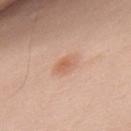subject: male, about 70 years old | lighting: white-light | location: the upper back | lesion size: about 3 mm | image source: 15 mm crop, total-body photography | image-analysis metrics: an area of roughly 4.5 mm², an outline eccentricity of about 0.75 (0 = round, 1 = elongated), and a symmetry-axis asymmetry near 0.2; a border-irregularity index near 2/10 and internal color variation of about 2.5 on a 0–10 scale.